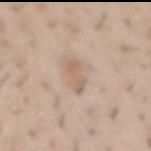Captured during whole-body skin photography for melanoma surveillance; the lesion was not biopsied. Imaged with white-light lighting. Located on the front of the torso. Cropped from a total-body skin-imaging series; the visible field is about 15 mm. A male subject about 30 years old.